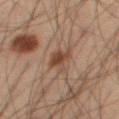follow-up = imaged on a skin check; not biopsied
size = about 3 mm
subject = male, about 55 years old
body site = the leg
image source = ~15 mm tile from a whole-body skin photo